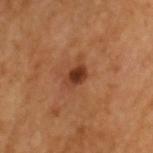Part of a total-body skin-imaging series; this lesion was reviewed on a skin check and was not flagged for biopsy.
On the head or neck.
A 15 mm crop from a total-body photograph taken for skin-cancer surveillance.
Imaged with cross-polarized lighting.
Longest diameter approximately 2.5 mm.
A male patient, aged approximately 45.
Automated image analysis of the tile measured an eccentricity of roughly 0.75 and two-axis asymmetry of about 0.15. And it measured an average lesion color of about L≈37 a*≈24 b*≈33 (CIELAB) and roughly 12 lightness units darker than nearby skin. And it measured a detector confidence of about 100 out of 100 that the crop contains a lesion.